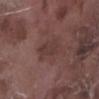Assessment: No biopsy was performed on this lesion — it was imaged during a full skin examination and was not determined to be concerning. Clinical summary: A male subject in their mid- to late 70s. Located on the right lower leg. Measured at roughly 3 mm in maximum diameter. This is a white-light tile. Automated tile analysis of the lesion measured an area of roughly 4.5 mm², a shape eccentricity near 0.85, and a shape-asymmetry score of about 0.25 (0 = symmetric). A 15 mm close-up tile from a total-body photography series done for melanoma screening.